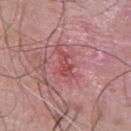notes = imaged on a skin check; not biopsied
location = the upper back
subject = male, roughly 45 years of age
automated metrics = a mean CIELAB color near L≈49 a*≈32 b*≈22, roughly 8 lightness units darker than nearby skin, and a normalized border contrast of about 6.5; a detector confidence of about 90 out of 100 that the crop contains a lesion
image = total-body-photography crop, ~15 mm field of view
diameter = about 3 mm
lighting = white-light illumination